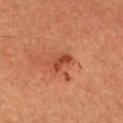follow-up: no biopsy performed (imaged during a skin exam) | image source: ~15 mm tile from a whole-body skin photo | subject: male, in their 60s.